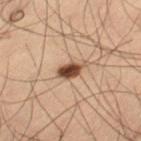{
  "biopsy_status": "not biopsied; imaged during a skin examination",
  "site": "left thigh",
  "lesion_size": {
    "long_diameter_mm_approx": 3.0
  },
  "lighting": "cross-polarized",
  "image": {
    "source": "total-body photography crop",
    "field_of_view_mm": 15
  },
  "automated_metrics": {
    "cielab_L": 38,
    "cielab_a": 16,
    "cielab_b": 25,
    "vs_skin_darker_L": 16.0,
    "vs_skin_contrast_norm": 12.5,
    "border_irregularity_0_10": 3.0,
    "peripheral_color_asymmetry": 1.5
  },
  "patient": {
    "sex": "male",
    "age_approx": 55
  }
}